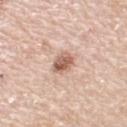illumination: white-light
automated metrics: a nevus-likeness score of about 90/100 and lesion-presence confidence of about 100/100
imaging modality: ~15 mm tile from a whole-body skin photo
subject: female, roughly 50 years of age
anatomic site: the arm
lesion size: ~2.5 mm (longest diameter)